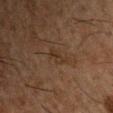subject: male, approximately 50 years of age
lesion size: ~3 mm (longest diameter)
lighting: cross-polarized
automated metrics: an area of roughly 3 mm², an eccentricity of roughly 0.9, and a shape-asymmetry score of about 0.3 (0 = symmetric); an average lesion color of about L≈24 a*≈13 b*≈22 (CIELAB), a lesion–skin lightness drop of about 4, and a lesion-to-skin contrast of about 5.5 (normalized; higher = more distinct); a border-irregularity index near 3/10, a within-lesion color-variation index near 1.5/10, and radial color variation of about 0.5; a classifier nevus-likeness of about 0/100 and a lesion-detection confidence of about 90/100
body site: the chest
imaging modality: 15 mm crop, total-body photography